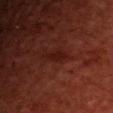{
  "biopsy_status": "not biopsied; imaged during a skin examination",
  "lighting": "cross-polarized",
  "lesion_size": {
    "long_diameter_mm_approx": 3.0
  },
  "automated_metrics": {
    "cielab_L": 17,
    "cielab_a": 24,
    "cielab_b": 22,
    "vs_skin_darker_L": 5.0,
    "vs_skin_contrast_norm": 7.0,
    "border_irregularity_0_10": 3.0,
    "color_variation_0_10": 0.0,
    "peripheral_color_asymmetry": 0.0
  },
  "image": {
    "source": "total-body photography crop",
    "field_of_view_mm": 15
  },
  "patient": {
    "sex": "male",
    "age_approx": 60
  },
  "site": "head or neck"
}